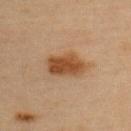{"biopsy_status": "not biopsied; imaged during a skin examination", "patient": {"sex": "female", "age_approx": 45}, "lighting": "cross-polarized", "lesion_size": {"long_diameter_mm_approx": 4.5}, "site": "upper back", "image": {"source": "total-body photography crop", "field_of_view_mm": 15}}